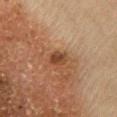notes=catalogued during a skin exam; not biopsied | image=~15 mm tile from a whole-body skin photo | location=the chest | patient=female, aged approximately 65 | diameter=~2.5 mm (longest diameter).